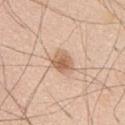Part of a total-body skin-imaging series; this lesion was reviewed on a skin check and was not flagged for biopsy. The subject is a male roughly 60 years of age. A region of skin cropped from a whole-body photographic capture, roughly 15 mm wide. The lesion's longest dimension is about 3 mm. The lesion is located on the abdomen.